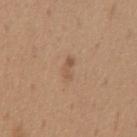| feature | finding |
|---|---|
| follow-up | imaged on a skin check; not biopsied |
| patient | male, in their mid-60s |
| image source | total-body-photography crop, ~15 mm field of view |
| site | the chest |